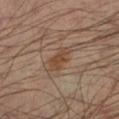Case summary:
* workup: no biopsy performed (imaged during a skin exam)
* image-analysis metrics: a footprint of about 5 mm² and a symmetry-axis asymmetry near 0.3; a lesion color around L≈41 a*≈17 b*≈28 in CIELAB, about 7 CIELAB-L* units darker than the surrounding skin, and a normalized border contrast of about 7
* anatomic site: the leg
* patient: male, in their mid- to late 40s
* image: ~15 mm crop, total-body skin-cancer survey
* diameter: ≈3.5 mm
* illumination: cross-polarized